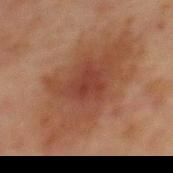{"biopsy_status": "not biopsied; imaged during a skin examination", "image": {"source": "total-body photography crop", "field_of_view_mm": 15}, "lesion_size": {"long_diameter_mm_approx": 12.0}, "site": "chest", "patient": {"sex": "male", "age_approx": 65}}